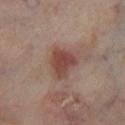Part of a total-body skin-imaging series; this lesion was reviewed on a skin check and was not flagged for biopsy.
An algorithmic analysis of the crop reported an area of roughly 8.5 mm², an outline eccentricity of about 0.6 (0 = round, 1 = elongated), and two-axis asymmetry of about 0.4. And it measured a border-irregularity index near 3.5/10, internal color variation of about 3.5 on a 0–10 scale, and a peripheral color-asymmetry measure near 1. And it measured a classifier nevus-likeness of about 75/100 and a lesion-detection confidence of about 100/100.
This is a cross-polarized tile.
The lesion is located on the left lower leg.
A 15 mm close-up tile from a total-body photography series done for melanoma screening.
Approximately 4 mm at its widest.
A male subject, about 70 years old.